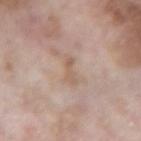– notes — catalogued during a skin exam; not biopsied
– anatomic site — the left forearm
– diameter — about 3 mm
– image source — total-body-photography crop, ~15 mm field of view
– illumination — white-light illumination
– patient — male, approximately 60 years of age